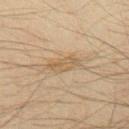Case summary:
– workup: imaged on a skin check; not biopsied
– site: the mid back
– acquisition: 15 mm crop, total-body photography
– size: ≈4.5 mm
– patient: male, in their mid- to late 30s